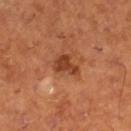follow-up: total-body-photography surveillance lesion; no biopsy | anatomic site: the right lower leg | image-analysis metrics: a lesion area of about 5.5 mm², a shape eccentricity near 0.75, and a symmetry-axis asymmetry near 0.4; a mean CIELAB color near L≈42 a*≈28 b*≈36, about 12 CIELAB-L* units darker than the surrounding skin, and a lesion-to-skin contrast of about 8.5 (normalized; higher = more distinct); border irregularity of about 4 on a 0–10 scale, a color-variation rating of about 2/10, and peripheral color asymmetry of about 0.5; a detector confidence of about 100 out of 100 that the crop contains a lesion | acquisition: ~15 mm crop, total-body skin-cancer survey | subject: male, aged approximately 65 | tile lighting: cross-polarized | lesion diameter: ~3.5 mm (longest diameter).